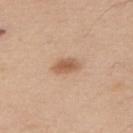lesion diameter: about 3.5 mm | tile lighting: white-light | acquisition: ~15 mm tile from a whole-body skin photo | automated metrics: a lesion area of about 5.5 mm², an outline eccentricity of about 0.8 (0 = round, 1 = elongated), and two-axis asymmetry of about 0.15; a nevus-likeness score of about 95/100 and lesion-presence confidence of about 100/100 | subject: male, approximately 55 years of age | anatomic site: the back.